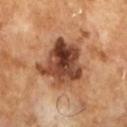- size — ~6.5 mm (longest diameter)
- patient — male, aged approximately 65
- lighting — cross-polarized
- image — total-body-photography crop, ~15 mm field of view
- image-analysis metrics — an outline eccentricity of about 0.4 (0 = round, 1 = elongated) and two-axis asymmetry of about 0.35; an average lesion color of about L≈44 a*≈23 b*≈31 (CIELAB) and about 17 CIELAB-L* units darker than the surrounding skin; peripheral color asymmetry of about 3.5; a nevus-likeness score of about 15/100 and a detector confidence of about 100 out of 100 that the crop contains a lesion